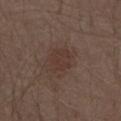Clinical impression:
Imaged during a routine full-body skin examination; the lesion was not biopsied and no histopathology is available.
Context:
This is a white-light tile. Longest diameter approximately 3 mm. On the abdomen. A 15 mm close-up extracted from a 3D total-body photography capture. A male subject aged 68–72. The total-body-photography lesion software estimated an outline eccentricity of about 0.5 (0 = round, 1 = elongated). And it measured an average lesion color of about L≈35 a*≈16 b*≈22 (CIELAB). The analysis additionally found border irregularity of about 2 on a 0–10 scale, a within-lesion color-variation index near 2/10, and radial color variation of about 0.5. And it measured an automated nevus-likeness rating near 15 out of 100 and lesion-presence confidence of about 100/100.